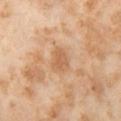Assessment: Imaged during a routine full-body skin examination; the lesion was not biopsied and no histopathology is available. Background: The lesion-visualizer software estimated a footprint of about 5.5 mm², an outline eccentricity of about 0.7 (0 = round, 1 = elongated), and a symmetry-axis asymmetry near 0.25. The analysis additionally found a border-irregularity index near 2/10, internal color variation of about 2 on a 0–10 scale, and peripheral color asymmetry of about 0.5. And it measured a nevus-likeness score of about 0/100 and a detector confidence of about 100 out of 100 that the crop contains a lesion. A female patient aged around 55. From the left thigh. A 15 mm close-up tile from a total-body photography series done for melanoma screening.